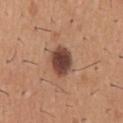Imaged during a routine full-body skin examination; the lesion was not biopsied and no histopathology is available. A male subject, approximately 40 years of age. This image is a 15 mm lesion crop taken from a total-body photograph. From the mid back.